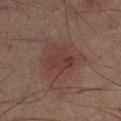Clinical summary: Captured under cross-polarized illumination. A male patient, about 50 years old. Approximately 6 mm at its widest. A lesion tile, about 15 mm wide, cut from a 3D total-body photograph. The lesion is on the left lower leg. The total-body-photography lesion software estimated an area of roughly 18 mm², an outline eccentricity of about 0.65 (0 = round, 1 = elongated), and a shape-asymmetry score of about 0.35 (0 = symmetric). It also reported border irregularity of about 4 on a 0–10 scale, a within-lesion color-variation index near 3/10, and a peripheral color-asymmetry measure near 1.